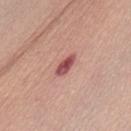Q: Automated lesion metrics?
A: a footprint of about 4 mm², an eccentricity of roughly 0.9, and a shape-asymmetry score of about 0.2 (0 = symmetric); about 15 CIELAB-L* units darker than the surrounding skin and a normalized border contrast of about 10.5
Q: Patient demographics?
A: female, in their mid-60s
Q: What is the lesion's diameter?
A: about 3.5 mm
Q: Lesion location?
A: the front of the torso
Q: How was this image acquired?
A: 15 mm crop, total-body photography